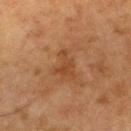  site: right thigh
  image:
    source: total-body photography crop
    field_of_view_mm: 15
  patient:
    sex: male
    age_approx: 80